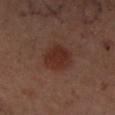| field | value |
|---|---|
| workup | total-body-photography surveillance lesion; no biopsy |
| image source | ~15 mm crop, total-body skin-cancer survey |
| subject | female, about 40 years old |
| illumination | cross-polarized |
| image-analysis metrics | a footprint of about 11 mm², a shape eccentricity near 0.55, and two-axis asymmetry of about 0.15; a nevus-likeness score of about 100/100 and lesion-presence confidence of about 100/100 |
| body site | the arm |